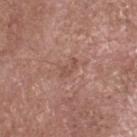Captured during whole-body skin photography for melanoma surveillance; the lesion was not biopsied. Automated image analysis of the tile measured a lesion area of about 2.5 mm², a shape eccentricity near 0.85, and a shape-asymmetry score of about 0.45 (0 = symmetric). The analysis additionally found a lesion color around L≈51 a*≈21 b*≈26 in CIELAB and a lesion–skin lightness drop of about 7. The analysis additionally found a border-irregularity index near 5.5/10, a color-variation rating of about 0/10, and peripheral color asymmetry of about 0. It also reported a nevus-likeness score of about 0/100 and a lesion-detection confidence of about 100/100. The lesion is on the head or neck. This is a white-light tile. A male subject, about 75 years old. A roughly 15 mm field-of-view crop from a total-body skin photograph. The lesion's longest dimension is about 2.5 mm.